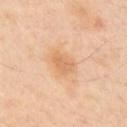Assessment: The lesion was tiled from a total-body skin photograph and was not biopsied. Context: A 15 mm close-up extracted from a 3D total-body photography capture. The lesion is on the right upper arm. Automated image analysis of the tile measured a lesion area of about 6 mm² and a shape eccentricity near 0.75. The software also gave a classifier nevus-likeness of about 15/100. The recorded lesion diameter is about 3.5 mm. This is a cross-polarized tile. The subject is a male aged around 50.